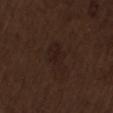Captured during whole-body skin photography for melanoma surveillance; the lesion was not biopsied.
The lesion is located on the back.
The lesion-visualizer software estimated a footprint of about 6.5 mm², an eccentricity of roughly 0.85, and two-axis asymmetry of about 0.35. And it measured a mean CIELAB color near L≈18 a*≈14 b*≈17.
The subject is a male in their 70s.
This image is a 15 mm lesion crop taken from a total-body photograph.